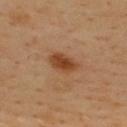Impression:
The lesion was tiled from a total-body skin photograph and was not biopsied.
Acquisition and patient details:
Captured under cross-polarized illumination. The total-body-photography lesion software estimated a detector confidence of about 100 out of 100 that the crop contains a lesion. The lesion is on the upper back. Cropped from a whole-body photographic skin survey; the tile spans about 15 mm. The patient is a male in their 40s. About 3.5 mm across.